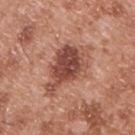The lesion was tiled from a total-body skin photograph and was not biopsied. Automated image analysis of the tile measured a border-irregularity rating of about 5/10 and peripheral color asymmetry of about 1.5. The analysis additionally found a classifier nevus-likeness of about 60/100 and a detector confidence of about 100 out of 100 that the crop contains a lesion. A roughly 15 mm field-of-view crop from a total-body skin photograph. The lesion is on the back. This is a white-light tile. A male subject, in their mid- to late 50s. Longest diameter approximately 6 mm.